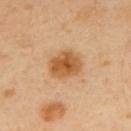Impression: No biopsy was performed on this lesion — it was imaged during a full skin examination and was not determined to be concerning. Clinical summary: Imaged with cross-polarized lighting. Cropped from a total-body skin-imaging series; the visible field is about 15 mm. The lesion is on the upper back. The subject is a male approximately 40 years of age. Automated image analysis of the tile measured a footprint of about 9.5 mm², an outline eccentricity of about 0.55 (0 = round, 1 = elongated), and a shape-asymmetry score of about 0.15 (0 = symmetric). The software also gave an average lesion color of about L≈56 a*≈23 b*≈42 (CIELAB), a lesion–skin lightness drop of about 12, and a normalized border contrast of about 9.5. The analysis additionally found a border-irregularity rating of about 1.5/10 and a color-variation rating of about 4.5/10. It also reported a nevus-likeness score of about 100/100. The recorded lesion diameter is about 4 mm.